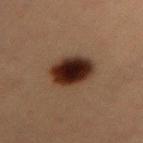This lesion was catalogued during total-body skin photography and was not selected for biopsy.